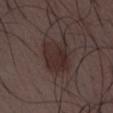{"biopsy_status": "not biopsied; imaged during a skin examination", "patient": {"sex": "male", "age_approx": 30}, "image": {"source": "total-body photography crop", "field_of_view_mm": 15}, "site": "front of the torso"}